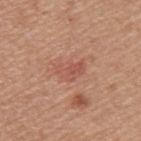biopsy status = catalogued during a skin exam; not biopsied
site = the upper back
automated metrics = a footprint of about 5.5 mm², a shape eccentricity near 0.8, and two-axis asymmetry of about 0.35; a mean CIELAB color near L≈54 a*≈26 b*≈29, roughly 7 lightness units darker than nearby skin, and a normalized border contrast of about 5; a border-irregularity index near 3.5/10 and a color-variation rating of about 3/10; an automated nevus-likeness rating near 0 out of 100 and a lesion-detection confidence of about 100/100
lesion diameter = about 3.5 mm
image source = ~15 mm crop, total-body skin-cancer survey
patient = male, approximately 55 years of age